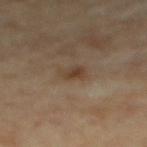This image is a 15 mm lesion crop taken from a total-body photograph. The tile uses cross-polarized illumination. The patient is a male aged 83 or older. Automated tile analysis of the lesion measured an average lesion color of about L≈39 a*≈14 b*≈26 (CIELAB), about 7 CIELAB-L* units darker than the surrounding skin, and a normalized border contrast of about 7. It also reported an automated nevus-likeness rating near 0 out of 100 and a lesion-detection confidence of about 100/100. The lesion is located on the upper back. Longest diameter approximately 3 mm.